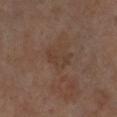This lesion was catalogued during total-body skin photography and was not selected for biopsy. A male subject aged 63–67. Cropped from a total-body skin-imaging series; the visible field is about 15 mm. On the left lower leg. This is a cross-polarized tile. About 3.5 mm across.